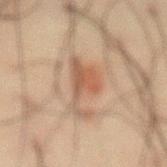The lesion was photographed on a routine skin check and not biopsied; there is no pathology result. The subject is a male aged approximately 60. Located on the abdomen. The lesion's longest dimension is about 6.5 mm. This is a cross-polarized tile. A roughly 15 mm field-of-view crop from a total-body skin photograph.